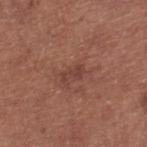No biopsy was performed on this lesion — it was imaged during a full skin examination and was not determined to be concerning. This is a white-light tile. Cropped from a whole-body photographic skin survey; the tile spans about 15 mm. A male subject, roughly 70 years of age. Automated tile analysis of the lesion measured an area of roughly 3 mm². Longest diameter approximately 2.5 mm. Located on the right lower leg.